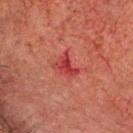| key | value |
|---|---|
| follow-up | total-body-photography surveillance lesion; no biopsy |
| patient | male, in their 60s |
| automated metrics | an outline eccentricity of about 0.7 (0 = round, 1 = elongated) and a shape-asymmetry score of about 0.5 (0 = symmetric); a lesion-detection confidence of about 100/100 |
| image source | ~15 mm crop, total-body skin-cancer survey |
| anatomic site | the head or neck |
| diameter | ~3 mm (longest diameter) |
| tile lighting | cross-polarized |